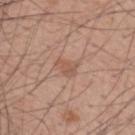workup: total-body-photography surveillance lesion; no biopsy
acquisition: 15 mm crop, total-body photography
patient: male, roughly 60 years of age
automated metrics: an average lesion color of about L≈54 a*≈21 b*≈29 (CIELAB), about 8 CIELAB-L* units darker than the surrounding skin, and a normalized border contrast of about 5.5; a border-irregularity index near 5/10, internal color variation of about 1 on a 0–10 scale, and radial color variation of about 0.5; an automated nevus-likeness rating near 0 out of 100 and a detector confidence of about 100 out of 100 that the crop contains a lesion
lesion size: ≈3 mm
anatomic site: the mid back
lighting: white-light illumination